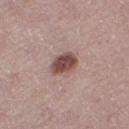Assessment: Part of a total-body skin-imaging series; this lesion was reviewed on a skin check and was not flagged for biopsy. Clinical summary: The subject is a female about 50 years old. On the right thigh. Automated image analysis of the tile measured an area of roughly 7.5 mm², an eccentricity of roughly 0.7, and a symmetry-axis asymmetry near 0.2. The software also gave a lesion–skin lightness drop of about 15 and a normalized border contrast of about 10.5. And it measured a border-irregularity rating of about 1.5/10, a color-variation rating of about 5/10, and radial color variation of about 2. The software also gave an automated nevus-likeness rating near 85 out of 100 and a detector confidence of about 100 out of 100 that the crop contains a lesion. Longest diameter approximately 3.5 mm. A roughly 15 mm field-of-view crop from a total-body skin photograph. Imaged with white-light lighting.